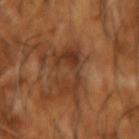Impression:
Recorded during total-body skin imaging; not selected for excision or biopsy.
Background:
This image is a 15 mm lesion crop taken from a total-body photograph. The recorded lesion diameter is about 5 mm. A male patient in their mid- to late 60s. From the left forearm.